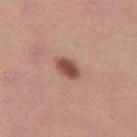follow-up — imaged on a skin check; not biopsied | illumination — white-light | site — the leg | patient — female, about 45 years old | image — 15 mm crop, total-body photography.